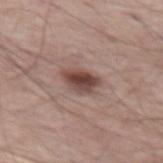- follow-up — catalogued during a skin exam; not biopsied
- image source — 15 mm crop, total-body photography
- anatomic site — the left thigh
- image-analysis metrics — a border-irregularity rating of about 2/10, a within-lesion color-variation index near 6/10, and radial color variation of about 1.5; a classifier nevus-likeness of about 95/100 and lesion-presence confidence of about 100/100
- illumination — white-light
- subject — male, roughly 65 years of age